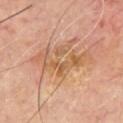follow-up: catalogued during a skin exam; not biopsied | lesion diameter: about 7 mm | patient: male, aged approximately 65 | body site: the chest | imaging modality: total-body-photography crop, ~15 mm field of view.